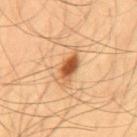The lesion is located on the mid back.
About 4.5 mm across.
The subject is a male in their mid-50s.
Captured under cross-polarized illumination.
The lesion-visualizer software estimated a mean CIELAB color near L≈58 a*≈24 b*≈40 and a lesion-to-skin contrast of about 9 (normalized; higher = more distinct).
A close-up tile cropped from a whole-body skin photograph, about 15 mm across.